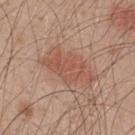workup: imaged on a skin check; not biopsied
image-analysis metrics: a lesion area of about 14 mm², an outline eccentricity of about 0.85 (0 = round, 1 = elongated), and two-axis asymmetry of about 0.3; a border-irregularity rating of about 5/10, a within-lesion color-variation index near 2.5/10, and peripheral color asymmetry of about 1; an automated nevus-likeness rating near 90 out of 100
site: the upper back
lighting: white-light illumination
acquisition: total-body-photography crop, ~15 mm field of view
subject: male, about 50 years old
lesion diameter: ≈6 mm